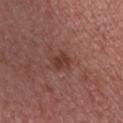  lighting: white-light
  image:
    source: total-body photography crop
    field_of_view_mm: 15
  lesion_size:
    long_diameter_mm_approx: 2.5
  patient:
    sex: male
    age_approx: 30
  automated_metrics:
    area_mm2_approx: 4.0
    eccentricity: 0.65
    shape_asymmetry: 0.25
    border_irregularity_0_10: 2.0
    color_variation_0_10: 1.5
    peripheral_color_asymmetry: 0.5